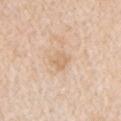* biopsy status · imaged on a skin check; not biopsied
* anatomic site · the left upper arm
* subject · female, approximately 45 years of age
* imaging modality · total-body-photography crop, ~15 mm field of view
* TBP lesion metrics · a border-irregularity index near 1.5/10, a color-variation rating of about 2/10, and a peripheral color-asymmetry measure near 0.5
* size · about 2.5 mm